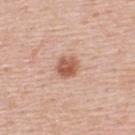biopsy status=imaged on a skin check; not biopsied | tile lighting=white-light | site=the back | subject=male, approximately 55 years of age | automated metrics=a lesion area of about 5.5 mm² and a symmetry-axis asymmetry near 0.15 | diameter=~3 mm (longest diameter) | imaging modality=~15 mm tile from a whole-body skin photo.